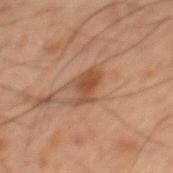| feature | finding |
|---|---|
| follow-up | no biopsy performed (imaged during a skin exam) |
| subject | male, in their 60s |
| image-analysis metrics | a mean CIELAB color near L≈37 a*≈17 b*≈26, a lesion–skin lightness drop of about 8, and a lesion-to-skin contrast of about 7 (normalized; higher = more distinct); an automated nevus-likeness rating near 100 out of 100 and lesion-presence confidence of about 100/100 |
| body site | the mid back |
| lighting | cross-polarized |
| acquisition | ~15 mm tile from a whole-body skin photo |